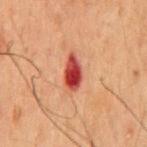Impression: Part of a total-body skin-imaging series; this lesion was reviewed on a skin check and was not flagged for biopsy. Acquisition and patient details: The recorded lesion diameter is about 4 mm. Automated tile analysis of the lesion measured a footprint of about 6 mm² and a symmetry-axis asymmetry near 0.15. And it measured an average lesion color of about L≈35 a*≈31 b*≈27 (CIELAB) and a normalized lesion–skin contrast near 12.5. The software also gave border irregularity of about 1.5 on a 0–10 scale and a peripheral color-asymmetry measure near 2. The tile uses cross-polarized illumination. On the chest. A region of skin cropped from a whole-body photographic capture, roughly 15 mm wide. A male patient approximately 50 years of age.